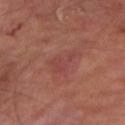Clinical impression: This lesion was catalogued during total-body skin photography and was not selected for biopsy. Acquisition and patient details: A male patient, approximately 70 years of age. On the left lower leg. A region of skin cropped from a whole-body photographic capture, roughly 15 mm wide.